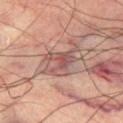Captured during whole-body skin photography for melanoma surveillance; the lesion was not biopsied. Cropped from a whole-body photographic skin survey; the tile spans about 15 mm. A male subject approximately 65 years of age. The lesion's longest dimension is about 5.5 mm. The tile uses cross-polarized illumination. The lesion is on the right thigh.